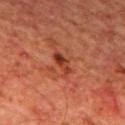Assessment:
Captured during whole-body skin photography for melanoma surveillance; the lesion was not biopsied.
Context:
Automated tile analysis of the lesion measured a footprint of about 3.5 mm², an outline eccentricity of about 0.9 (0 = round, 1 = elongated), and a symmetry-axis asymmetry near 0.25. The software also gave a mean CIELAB color near L≈35 a*≈29 b*≈32, about 10 CIELAB-L* units darker than the surrounding skin, and a normalized border contrast of about 8.5. The analysis additionally found a border-irregularity index near 3/10 and peripheral color asymmetry of about 0.5. From the mid back. A lesion tile, about 15 mm wide, cut from a 3D total-body photograph. Longest diameter approximately 3 mm. The tile uses cross-polarized illumination. A male patient, aged 63–67.